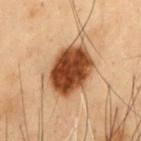Assessment:
Captured during whole-body skin photography for melanoma surveillance; the lesion was not biopsied.
Background:
A male patient, roughly 55 years of age. The lesion is located on the chest. Cropped from a total-body skin-imaging series; the visible field is about 15 mm.